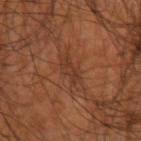The lesion was tiled from a total-body skin photograph and was not biopsied. About 3.5 mm across. Automated tile analysis of the lesion measured lesion-presence confidence of about 95/100. Captured under cross-polarized illumination. A close-up tile cropped from a whole-body skin photograph, about 15 mm across. A male patient aged around 45. On the right upper arm.